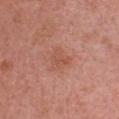notes = imaged on a skin check; not biopsied
illumination = white-light illumination
body site = the chest
acquisition = total-body-photography crop, ~15 mm field of view
subject = female, aged around 65
diameter = ~2.5 mm (longest diameter)